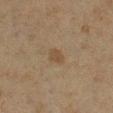| feature | finding |
|---|---|
| follow-up | total-body-photography surveillance lesion; no biopsy |
| lighting | cross-polarized illumination |
| imaging modality | 15 mm crop, total-body photography |
| anatomic site | the right lower leg |
| diameter | ≈2.5 mm |
| automated lesion analysis | a lesion color around L≈39 a*≈12 b*≈27 in CIELAB, a lesion–skin lightness drop of about 6, and a normalized border contrast of about 6 |
| patient | female, roughly 55 years of age |